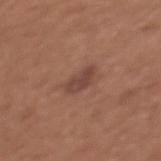Case summary:
* workup · total-body-photography surveillance lesion; no biopsy
* patient · female, aged approximately 35
* size · ~3.5 mm (longest diameter)
* anatomic site · the chest
* lighting · white-light illumination
* TBP lesion metrics · an area of roughly 5 mm², an outline eccentricity of about 0.8 (0 = round, 1 = elongated), and a shape-asymmetry score of about 0.25 (0 = symmetric); a border-irregularity rating of about 2.5/10, a within-lesion color-variation index near 2.5/10, and peripheral color asymmetry of about 1
* imaging modality · ~15 mm crop, total-body skin-cancer survey